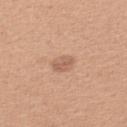workup: catalogued during a skin exam; not biopsied | lesion size: ~2.5 mm (longest diameter) | tile lighting: white-light illumination | subject: female, aged 38–42 | TBP lesion metrics: a footprint of about 4 mm², an outline eccentricity of about 0.65 (0 = round, 1 = elongated), and a shape-asymmetry score of about 0.15 (0 = symmetric); a mean CIELAB color near L≈60 a*≈21 b*≈30, about 9 CIELAB-L* units darker than the surrounding skin, and a lesion-to-skin contrast of about 6 (normalized; higher = more distinct); internal color variation of about 2 on a 0–10 scale and a peripheral color-asymmetry measure near 0.5; a nevus-likeness score of about 20/100 and a lesion-detection confidence of about 100/100 | location: the left upper arm | imaging modality: ~15 mm crop, total-body skin-cancer survey.